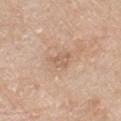{"biopsy_status": "not biopsied; imaged during a skin examination", "lighting": "white-light", "image": {"source": "total-body photography crop", "field_of_view_mm": 15}, "patient": {"sex": "male", "age_approx": 80}, "site": "front of the torso", "lesion_size": {"long_diameter_mm_approx": 2.5}, "automated_metrics": {"area_mm2_approx": 4.0, "eccentricity": 0.65}}